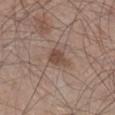Q: Was a biopsy performed?
A: total-body-photography surveillance lesion; no biopsy
Q: What is the imaging modality?
A: total-body-photography crop, ~15 mm field of view
Q: What is the anatomic site?
A: the right lower leg
Q: What are the patient's age and sex?
A: male, aged around 45